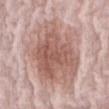{
  "biopsy_status": "not biopsied; imaged during a skin examination",
  "site": "front of the torso",
  "image": {
    "source": "total-body photography crop",
    "field_of_view_mm": 15
  },
  "automated_metrics": {
    "cielab_L": 59,
    "cielab_a": 20,
    "cielab_b": 24,
    "vs_skin_contrast_norm": 7.5,
    "border_irregularity_0_10": 3.5,
    "peripheral_color_asymmetry": 1.5
  },
  "patient": {
    "sex": "female",
    "age_approx": 65
  },
  "lesion_size": {
    "long_diameter_mm_approx": 9.5
  }
}